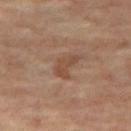biopsy status: total-body-photography surveillance lesion; no biopsy | imaging modality: total-body-photography crop, ~15 mm field of view | image-analysis metrics: an area of roughly 5 mm², an eccentricity of roughly 0.8, and two-axis asymmetry of about 0.5 | lighting: cross-polarized | subject: female, in their mid- to late 60s | body site: the right thigh.